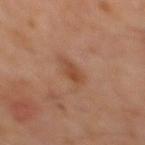Q: Was this lesion biopsied?
A: catalogued during a skin exam; not biopsied